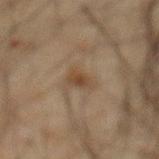No biopsy was performed on this lesion — it was imaged during a full skin examination and was not determined to be concerning.
The lesion is on the abdomen.
A male patient, about 65 years old.
The lesion-visualizer software estimated roughly 7 lightness units darker than nearby skin and a normalized border contrast of about 7. The analysis additionally found a border-irregularity rating of about 2.5/10 and a peripheral color-asymmetry measure near 1.
About 3 mm across.
This image is a 15 mm lesion crop taken from a total-body photograph.